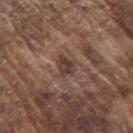workup: total-body-photography surveillance lesion; no biopsy
subject: male, approximately 75 years of age
imaging modality: ~15 mm tile from a whole-body skin photo
tile lighting: white-light
site: the right upper arm
automated metrics: a lesion–skin lightness drop of about 9